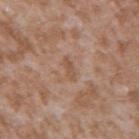Assessment: This lesion was catalogued during total-body skin photography and was not selected for biopsy. Image and clinical context: The lesion is located on the right upper arm. The patient is a male roughly 45 years of age. The tile uses white-light illumination. Longest diameter approximately 3 mm. A lesion tile, about 15 mm wide, cut from a 3D total-body photograph. An algorithmic analysis of the crop reported a lesion–skin lightness drop of about 7 and a lesion-to-skin contrast of about 6 (normalized; higher = more distinct). And it measured an automated nevus-likeness rating near 0 out of 100 and a detector confidence of about 100 out of 100 that the crop contains a lesion.